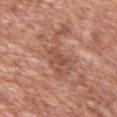biopsy_status: not biopsied; imaged during a skin examination
lesion_size:
  long_diameter_mm_approx: 3.0
site: front of the torso
automated_metrics:
  area_mm2_approx: 4.0
  shape_asymmetry: 0.4
  cielab_L: 50
  cielab_a: 24
  cielab_b: 30
  vs_skin_darker_L: 7.0
  vs_skin_contrast_norm: 5.5
  color_variation_0_10: 1.0
  peripheral_color_asymmetry: 0.5
  nevus_likeness_0_100: 0
  lesion_detection_confidence_0_100: 95
patient:
  sex: male
  age_approx: 50
lighting: white-light
image:
  source: total-body photography crop
  field_of_view_mm: 15